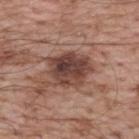Impression: The lesion was photographed on a routine skin check and not biopsied; there is no pathology result. Image and clinical context: An algorithmic analysis of the crop reported a footprint of about 15 mm² and a shape eccentricity near 0.6. The software also gave a lesion color around L≈42 a*≈20 b*≈23 in CIELAB, about 14 CIELAB-L* units darker than the surrounding skin, and a normalized lesion–skin contrast near 10.5. It also reported a color-variation rating of about 7/10 and peripheral color asymmetry of about 2.5. The software also gave a classifier nevus-likeness of about 35/100 and lesion-presence confidence of about 100/100. A male patient, aged approximately 70. A 15 mm close-up extracted from a 3D total-body photography capture. Longest diameter approximately 5 mm. The lesion is located on the upper back.